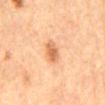workup = total-body-photography surveillance lesion; no biopsy
subject = male, aged 83 or older
automated metrics = lesion-presence confidence of about 100/100
image source = 15 mm crop, total-body photography
lighting = cross-polarized illumination
anatomic site = the mid back